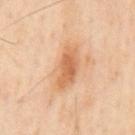Findings:
* workup · catalogued during a skin exam; not biopsied
* patient · male, aged around 55
* site · the mid back
* tile lighting · cross-polarized
* automated metrics · a footprint of about 12 mm² and a symmetry-axis asymmetry near 0.2; an average lesion color of about L≈66 a*≈23 b*≈38 (CIELAB), roughly 11 lightness units darker than nearby skin, and a normalized lesion–skin contrast near 7; a classifier nevus-likeness of about 90/100 and a detector confidence of about 100 out of 100 that the crop contains a lesion
* acquisition · ~15 mm crop, total-body skin-cancer survey